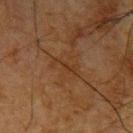The lesion was tiled from a total-body skin photograph and was not biopsied. A male subject about 65 years old. Longest diameter approximately 3.5 mm. The tile uses cross-polarized illumination. A 15 mm close-up extracted from a 3D total-body photography capture. On the left upper arm. Automated image analysis of the tile measured a footprint of about 3 mm², an outline eccentricity of about 0.95 (0 = round, 1 = elongated), and a shape-asymmetry score of about 0.5 (0 = symmetric). And it measured about 5 CIELAB-L* units darker than the surrounding skin and a normalized lesion–skin contrast near 5.5.